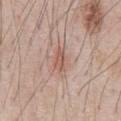No biopsy was performed on this lesion — it was imaged during a full skin examination and was not determined to be concerning. The lesion is on the chest. A male subject, about 70 years old. A close-up tile cropped from a whole-body skin photograph, about 15 mm across.